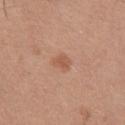Impression:
Recorded during total-body skin imaging; not selected for excision or biopsy.
Clinical summary:
The lesion is located on the front of the torso. Captured under white-light illumination. A male subject roughly 25 years of age. A roughly 15 mm field-of-view crop from a total-body skin photograph. Measured at roughly 2.5 mm in maximum diameter.